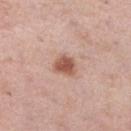Clinical impression: Captured during whole-body skin photography for melanoma surveillance; the lesion was not biopsied. Image and clinical context: Imaged with white-light lighting. Located on the right lower leg. The recorded lesion diameter is about 2.5 mm. An algorithmic analysis of the crop reported a footprint of about 5.5 mm², an eccentricity of roughly 0.5, and a symmetry-axis asymmetry near 0.2. The analysis additionally found border irregularity of about 1.5 on a 0–10 scale and radial color variation of about 0.5. It also reported an automated nevus-likeness rating near 95 out of 100 and lesion-presence confidence of about 100/100. A female patient, aged approximately 55. A region of skin cropped from a whole-body photographic capture, roughly 15 mm wide.